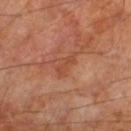This lesion was catalogued during total-body skin photography and was not selected for biopsy.
Cropped from a whole-body photographic skin survey; the tile spans about 15 mm.
The lesion is on the right lower leg.
The patient is a male aged 68–72.
Automated image analysis of the tile measured roughly 7 lightness units darker than nearby skin and a lesion-to-skin contrast of about 5.5 (normalized; higher = more distinct). And it measured lesion-presence confidence of about 100/100.